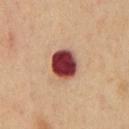biopsy status — no biopsy performed (imaged during a skin exam) | image source — ~15 mm crop, total-body skin-cancer survey | patient — male, approximately 60 years of age | TBP lesion metrics — a lesion area of about 11 mm², an outline eccentricity of about 0.3 (0 = round, 1 = elongated), and a symmetry-axis asymmetry near 0.15; an average lesion color of about L≈38 a*≈28 b*≈23 (CIELAB), roughly 24 lightness units darker than nearby skin, and a lesion-to-skin contrast of about 17.5 (normalized; higher = more distinct); border irregularity of about 1 on a 0–10 scale, internal color variation of about 6 on a 0–10 scale, and peripheral color asymmetry of about 1.5; a classifier nevus-likeness of about 0/100 and a detector confidence of about 100 out of 100 that the crop contains a lesion | lesion diameter — ≈3.5 mm | illumination — cross-polarized | site — the chest.